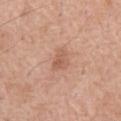Q: Was a biopsy performed?
A: no biopsy performed (imaged during a skin exam)
Q: What are the patient's age and sex?
A: male, aged 58–62
Q: Where on the body is the lesion?
A: the chest
Q: What kind of image is this?
A: total-body-photography crop, ~15 mm field of view
Q: What lighting was used for the tile?
A: white-light illumination
Q: How large is the lesion?
A: ≈3 mm